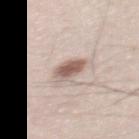follow-up=imaged on a skin check; not biopsied | site=the mid back | size=~3 mm (longest diameter) | subject=male, roughly 35 years of age | image-analysis metrics=a mean CIELAB color near L≈59 a*≈16 b*≈23; a peripheral color-asymmetry measure near 1; an automated nevus-likeness rating near 90 out of 100 and a detector confidence of about 100 out of 100 that the crop contains a lesion | lighting=white-light | image source=~15 mm crop, total-body skin-cancer survey.